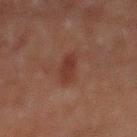Part of a total-body skin-imaging series; this lesion was reviewed on a skin check and was not flagged for biopsy.